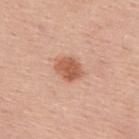This lesion was catalogued during total-body skin photography and was not selected for biopsy.
On the upper back.
The total-body-photography lesion software estimated a border-irregularity index near 1.5/10, a color-variation rating of about 3/10, and peripheral color asymmetry of about 1. And it measured a nevus-likeness score of about 100/100 and a lesion-detection confidence of about 100/100.
A 15 mm close-up extracted from a 3D total-body photography capture.
Measured at roughly 3.5 mm in maximum diameter.
This is a white-light tile.
The subject is a male aged 53–57.